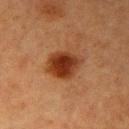A 15 mm close-up tile from a total-body photography series done for melanoma screening. Captured under cross-polarized illumination. The subject is a female aged 53–57. On the right upper arm.